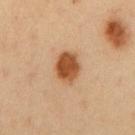workup — imaged on a skin check; not biopsied
size — ~3.5 mm (longest diameter)
image — ~15 mm crop, total-body skin-cancer survey
body site — the chest
subject — male, aged 48 to 52
illumination — cross-polarized
TBP lesion metrics — an area of roughly 8.5 mm² and a shape-asymmetry score of about 0.2 (0 = symmetric); roughly 16 lightness units darker than nearby skin and a lesion-to-skin contrast of about 11.5 (normalized; higher = more distinct)